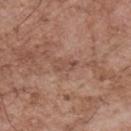biopsy status = catalogued during a skin exam; not biopsied